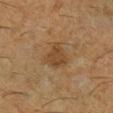Q: Was a biopsy performed?
A: total-body-photography surveillance lesion; no biopsy
Q: What did automated image analysis measure?
A: an area of roughly 7 mm², an eccentricity of roughly 0.65, and a symmetry-axis asymmetry near 0.35; a border-irregularity rating of about 3.5/10; a nevus-likeness score of about 20/100 and lesion-presence confidence of about 100/100
Q: Who is the patient?
A: male, aged around 60
Q: What is the lesion's diameter?
A: ≈4 mm
Q: What kind of image is this?
A: ~15 mm crop, total-body skin-cancer survey
Q: What is the anatomic site?
A: the right lower leg
Q: How was the tile lit?
A: cross-polarized illumination